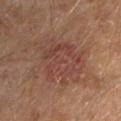Located on the left upper arm. The patient is a male roughly 65 years of age. A close-up tile cropped from a whole-body skin photograph, about 15 mm across.